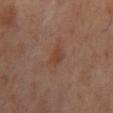Q: What did automated image analysis measure?
A: a lesion area of about 4 mm² and two-axis asymmetry of about 0.25
Q: How was the tile lit?
A: cross-polarized
Q: Where on the body is the lesion?
A: the back
Q: What is the imaging modality?
A: 15 mm crop, total-body photography
Q: What are the patient's age and sex?
A: male, aged 63 to 67